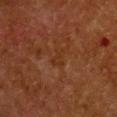The lesion was photographed on a routine skin check and not biopsied; there is no pathology result. A 15 mm close-up tile from a total-body photography series done for melanoma screening. A female subject, in their 50s. From the chest. About 2.5 mm across. Captured under cross-polarized illumination.